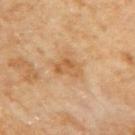No biopsy was performed on this lesion — it was imaged during a full skin examination and was not determined to be concerning.
The tile uses cross-polarized illumination.
The lesion is on the right upper arm.
The subject is a female about 60 years old.
A 15 mm close-up tile from a total-body photography series done for melanoma screening.